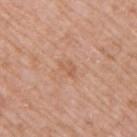* notes: total-body-photography surveillance lesion; no biopsy
* imaging modality: ~15 mm crop, total-body skin-cancer survey
* body site: the right upper arm
* patient: male, aged around 50